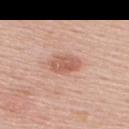This lesion was catalogued during total-body skin photography and was not selected for biopsy.
An algorithmic analysis of the crop reported a lesion area of about 6.5 mm², an outline eccentricity of about 0.8 (0 = round, 1 = elongated), and a symmetry-axis asymmetry near 0.15. The analysis additionally found radial color variation of about 1. And it measured a nevus-likeness score of about 65/100 and a lesion-detection confidence of about 100/100.
The lesion is located on the upper back.
A 15 mm close-up tile from a total-body photography series done for melanoma screening.
The recorded lesion diameter is about 3.5 mm.
A male subject in their mid- to late 50s.